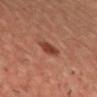No biopsy was performed on this lesion — it was imaged during a full skin examination and was not determined to be concerning.
This image is a 15 mm lesion crop taken from a total-body photograph.
The subject is a male in their mid- to late 30s.
The total-body-photography lesion software estimated roughly 11 lightness units darker than nearby skin. The analysis additionally found a nevus-likeness score of about 90/100 and lesion-presence confidence of about 100/100.
The lesion is on the chest.